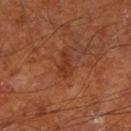Part of a total-body skin-imaging series; this lesion was reviewed on a skin check and was not flagged for biopsy. Imaged with cross-polarized lighting. The lesion-visualizer software estimated internal color variation of about 2 on a 0–10 scale and peripheral color asymmetry of about 0.5. The lesion's longest dimension is about 3 mm. The lesion is located on the leg. A 15 mm crop from a total-body photograph taken for skin-cancer surveillance. The patient is a male in their mid- to late 60s.